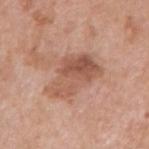Assessment:
No biopsy was performed on this lesion — it was imaged during a full skin examination and was not determined to be concerning.
Image and clinical context:
Imaged with white-light lighting. The recorded lesion diameter is about 5.5 mm. A male subject aged 58–62. A 15 mm close-up extracted from a 3D total-body photography capture. From the right upper arm.